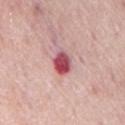patient: male, in their mid-60s | image-analysis metrics: a footprint of about 6 mm² and a shape-asymmetry score of about 0.2 (0 = symmetric); an average lesion color of about L≈53 a*≈33 b*≈20 (CIELAB), a lesion–skin lightness drop of about 18, and a lesion-to-skin contrast of about 11.5 (normalized; higher = more distinct); peripheral color asymmetry of about 2.5 | diameter: about 3.5 mm | lighting: white-light | image: ~15 mm tile from a whole-body skin photo | body site: the chest.